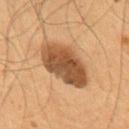Part of a total-body skin-imaging series; this lesion was reviewed on a skin check and was not flagged for biopsy. On the chest. This is a cross-polarized tile. A male subject roughly 55 years of age. Approximately 6.5 mm at its widest. A 15 mm close-up extracted from a 3D total-body photography capture.